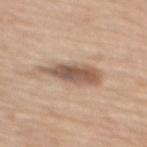Findings:
• workup · catalogued during a skin exam; not biopsied
• patient · female, about 70 years old
• image source · ~15 mm crop, total-body skin-cancer survey
• site · the mid back
• lighting · white-light illumination